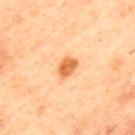Notes:
– image-analysis metrics: a lesion area of about 4 mm², an eccentricity of roughly 0.7, and a shape-asymmetry score of about 0.2 (0 = symmetric); a border-irregularity index near 2/10, a color-variation rating of about 3/10, and radial color variation of about 1
– site: the back
– image source: ~15 mm tile from a whole-body skin photo
– lighting: cross-polarized illumination
– patient: male, in their mid- to late 40s